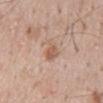Assessment: No biopsy was performed on this lesion — it was imaged during a full skin examination and was not determined to be concerning.